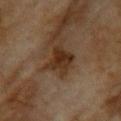{"biopsy_status": "not biopsied; imaged during a skin examination", "patient": {"sex": "female", "age_approx": 60}, "site": "upper back", "image": {"source": "total-body photography crop", "field_of_view_mm": 15}}